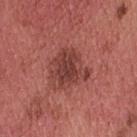Q: Was a biopsy performed?
A: catalogued during a skin exam; not biopsied
Q: What is the anatomic site?
A: the head or neck
Q: How was the tile lit?
A: white-light
Q: Patient demographics?
A: male, aged around 55
Q: What did automated image analysis measure?
A: a lesion area of about 14 mm², a shape eccentricity near 0.4, and a shape-asymmetry score of about 0.3 (0 = symmetric); an automated nevus-likeness rating near 35 out of 100 and lesion-presence confidence of about 100/100
Q: What is the lesion's diameter?
A: ≈4.5 mm
Q: What is the imaging modality?
A: ~15 mm tile from a whole-body skin photo